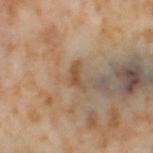  biopsy_status: not biopsied; imaged during a skin examination
  lighting: cross-polarized
  patient:
    sex: female
    age_approx: 55
  site: left thigh
  image:
    source: total-body photography crop
    field_of_view_mm: 15
  automated_metrics:
    area_mm2_approx: 3.0
    eccentricity: 0.9
    shape_asymmetry: 0.5
    cielab_L: 51
    cielab_a: 18
    cielab_b: 33
    vs_skin_darker_L: 9.0
    vs_skin_contrast_norm: 7.0
    nevus_likeness_0_100: 0
    lesion_detection_confidence_0_100: 100